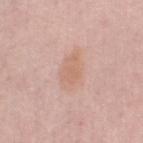The lesion is on the left lower leg.
A 15 mm crop from a total-body photograph taken for skin-cancer surveillance.
A male subject roughly 65 years of age.